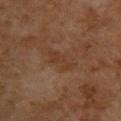{
  "biopsy_status": "not biopsied; imaged during a skin examination",
  "patient": {
    "sex": "female",
    "age_approx": 60
  },
  "image": {
    "source": "total-body photography crop",
    "field_of_view_mm": 15
  },
  "automated_metrics": {
    "area_mm2_approx": 6.0,
    "eccentricity": 0.9,
    "vs_skin_darker_L": 4.0,
    "vs_skin_contrast_norm": 5.0,
    "nevus_likeness_0_100": 0,
    "lesion_detection_confidence_0_100": 100
  },
  "site": "upper back",
  "lesion_size": {
    "long_diameter_mm_approx": 4.0
  }
}